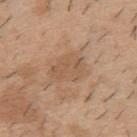Q: Is there a histopathology result?
A: catalogued during a skin exam; not biopsied
Q: Lesion size?
A: ~4 mm (longest diameter)
Q: Where on the body is the lesion?
A: the back
Q: Who is the patient?
A: male, aged 38 to 42
Q: How was this image acquired?
A: ~15 mm crop, total-body skin-cancer survey
Q: Automated lesion metrics?
A: a lesion area of about 9 mm² and two-axis asymmetry of about 0.3; a mean CIELAB color near L≈55 a*≈18 b*≈32, about 6 CIELAB-L* units darker than the surrounding skin, and a normalized lesion–skin contrast near 4.5; a color-variation rating of about 2.5/10 and radial color variation of about 1; a classifier nevus-likeness of about 0/100 and a detector confidence of about 100 out of 100 that the crop contains a lesion
Q: What lighting was used for the tile?
A: white-light illumination